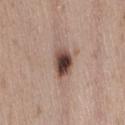No biopsy was performed on this lesion — it was imaged during a full skin examination and was not determined to be concerning. A 15 mm close-up tile from a total-body photography series done for melanoma screening. A female subject about 30 years old. The tile uses white-light illumination. Located on the back.